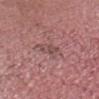<lesion>
<biopsy_status>not biopsied; imaged during a skin examination</biopsy_status>
<site>head or neck</site>
<patient>
  <sex>male</sex>
  <age_approx>75</age_approx>
</patient>
<image>
  <source>total-body photography crop</source>
  <field_of_view_mm>15</field_of_view_mm>
</image>
</lesion>